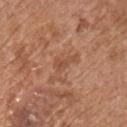Case summary:
- biopsy status — imaged on a skin check; not biopsied
- image source — ~15 mm tile from a whole-body skin photo
- subject — male, aged around 65
- anatomic site — the chest